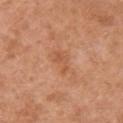{"site": "left arm", "lesion_size": {"long_diameter_mm_approx": 3.0}, "automated_metrics": {"area_mm2_approx": 3.0, "eccentricity": 0.85, "shape_asymmetry": 0.55, "border_irregularity_0_10": 6.5, "color_variation_0_10": 1.0, "peripheral_color_asymmetry": 0.5}, "lighting": "white-light", "patient": {"sex": "female", "age_approx": 65}, "image": {"source": "total-body photography crop", "field_of_view_mm": 15}}